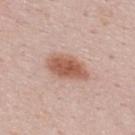<tbp_lesion>
<biopsy_status>not biopsied; imaged during a skin examination</biopsy_status>
<patient>
  <sex>male</sex>
  <age_approx>25</age_approx>
</patient>
<image>
  <source>total-body photography crop</source>
  <field_of_view_mm>15</field_of_view_mm>
</image>
<lighting>white-light</lighting>
<automated_metrics>
  <nevus_likeness_0_100>100</nevus_likeness_0_100>
  <lesion_detection_confidence_0_100>100</lesion_detection_confidence_0_100>
</automated_metrics>
<site>upper back</site>
</tbp_lesion>